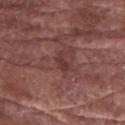Part of a total-body skin-imaging series; this lesion was reviewed on a skin check and was not flagged for biopsy.
A lesion tile, about 15 mm wide, cut from a 3D total-body photograph.
On the right forearm.
A male subject about 75 years old.